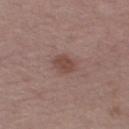Imaged during a routine full-body skin examination; the lesion was not biopsied and no histopathology is available. The subject is a female about 50 years old. A 15 mm close-up extracted from a 3D total-body photography capture. Located on the right thigh.